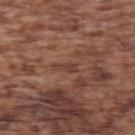No biopsy was performed on this lesion — it was imaged during a full skin examination and was not determined to be concerning. The lesion is located on the left upper arm. The recorded lesion diameter is about 2.5 mm. A roughly 15 mm field-of-view crop from a total-body skin photograph. Captured under white-light illumination. An algorithmic analysis of the crop reported an outline eccentricity of about 0.9 (0 = round, 1 = elongated) and two-axis asymmetry of about 0.45. A male subject in their mid-70s.